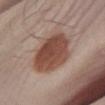Image and clinical context: On the right lower leg. A region of skin cropped from a whole-body photographic capture, roughly 15 mm wide. The subject is a male about 65 years old.